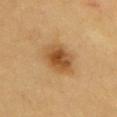Recorded during total-body skin imaging; not selected for excision or biopsy.
The patient is a male aged approximately 60.
Located on the chest.
The tile uses cross-polarized illumination.
A 15 mm close-up tile from a total-body photography series done for melanoma screening.